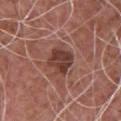Recorded during total-body skin imaging; not selected for excision or biopsy.
Automated image analysis of the tile measured a mean CIELAB color near L≈39 a*≈23 b*≈24, roughly 11 lightness units darker than nearby skin, and a lesion-to-skin contrast of about 9 (normalized; higher = more distinct). The software also gave a border-irregularity rating of about 2/10. And it measured a classifier nevus-likeness of about 5/100 and a lesion-detection confidence of about 100/100.
Located on the chest.
Longest diameter approximately 3 mm.
Captured under white-light illumination.
Cropped from a total-body skin-imaging series; the visible field is about 15 mm.
A male patient, aged approximately 75.